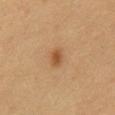Q: How was this image acquired?
A: ~15 mm crop, total-body skin-cancer survey
Q: What is the anatomic site?
A: the front of the torso
Q: Illumination type?
A: cross-polarized
Q: Patient demographics?
A: male, aged around 60